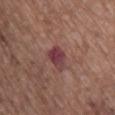Recorded during total-body skin imaging; not selected for excision or biopsy. Located on the upper back. This image is a 15 mm lesion crop taken from a total-body photograph. An algorithmic analysis of the crop reported a lesion area of about 5.5 mm², an eccentricity of roughly 0.65, and a shape-asymmetry score of about 0.3 (0 = symmetric). And it measured about 10 CIELAB-L* units darker than the surrounding skin and a lesion-to-skin contrast of about 10 (normalized; higher = more distinct). The analysis additionally found a border-irregularity rating of about 3/10, a within-lesion color-variation index near 4.5/10, and peripheral color asymmetry of about 1. The analysis additionally found a classifier nevus-likeness of about 0/100. The patient is a female aged 73–77. About 3 mm across. The tile uses white-light illumination.